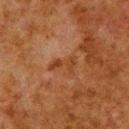| field | value |
|---|---|
| notes | imaged on a skin check; not biopsied |
| acquisition | ~15 mm crop, total-body skin-cancer survey |
| diameter | ≈3.5 mm |
| tile lighting | cross-polarized illumination |
| subject | male, aged 78 to 82 |
| location | the upper back |